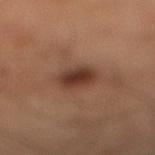This lesion was catalogued during total-body skin photography and was not selected for biopsy. Cropped from a total-body skin-imaging series; the visible field is about 15 mm. A male subject, aged 58–62. Captured under cross-polarized illumination. The total-body-photography lesion software estimated a footprint of about 7 mm² and a symmetry-axis asymmetry near 0.15. The software also gave an average lesion color of about L≈36 a*≈21 b*≈26 (CIELAB), about 11 CIELAB-L* units darker than the surrounding skin, and a lesion-to-skin contrast of about 10 (normalized; higher = more distinct). The software also gave an automated nevus-likeness rating near 95 out of 100 and a lesion-detection confidence of about 100/100. On the right lower leg.